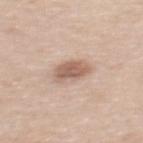This lesion was catalogued during total-body skin photography and was not selected for biopsy.
Longest diameter approximately 3.5 mm.
The tile uses white-light illumination.
A close-up tile cropped from a whole-body skin photograph, about 15 mm across.
An algorithmic analysis of the crop reported a footprint of about 7 mm² and a shape-asymmetry score of about 0.25 (0 = symmetric). It also reported a mean CIELAB color near L≈60 a*≈18 b*≈27, roughly 12 lightness units darker than nearby skin, and a normalized lesion–skin contrast near 8. The software also gave a lesion-detection confidence of about 100/100.
The lesion is located on the mid back.
The subject is a male roughly 50 years of age.